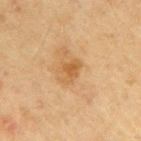workup = no biopsy performed (imaged during a skin exam) | image source = ~15 mm crop, total-body skin-cancer survey | anatomic site = the right upper arm | subject = male, aged 68–72 | size = about 2.5 mm.